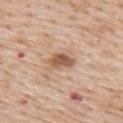Captured during whole-body skin photography for melanoma surveillance; the lesion was not biopsied. The recorded lesion diameter is about 3.5 mm. The lesion is on the upper back. A female patient roughly 70 years of age. Automated tile analysis of the lesion measured a lesion area of about 5.5 mm², a shape eccentricity near 0.8, and two-axis asymmetry of about 0.25. The analysis additionally found an average lesion color of about L≈57 a*≈20 b*≈32 (CIELAB) and a normalized border contrast of about 8.5. The analysis additionally found an automated nevus-likeness rating near 90 out of 100. The tile uses white-light illumination. Cropped from a total-body skin-imaging series; the visible field is about 15 mm.